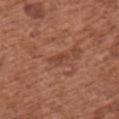No biopsy was performed on this lesion — it was imaged during a full skin examination and was not determined to be concerning. About 2.5 mm across. Imaged with white-light lighting. Cropped from a total-body skin-imaging series; the visible field is about 15 mm. A female subject, aged 73 to 77. The lesion is on the upper back.